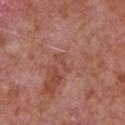patient — male, aged 63–67 | image — 15 mm crop, total-body photography | lighting — white-light illumination | automated lesion analysis — a within-lesion color-variation index near 0/10 | body site — the chest.